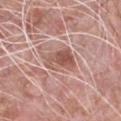This lesion was catalogued during total-body skin photography and was not selected for biopsy. A male patient, in their 50s. On the chest. Automated image analysis of the tile measured an automated nevus-likeness rating near 70 out of 100. A 15 mm close-up extracted from a 3D total-body photography capture.